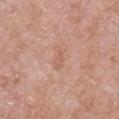Clinical impression:
No biopsy was performed on this lesion — it was imaged during a full skin examination and was not determined to be concerning.
Acquisition and patient details:
The subject is a male in their mid-60s. A region of skin cropped from a whole-body photographic capture, roughly 15 mm wide. Located on the right upper arm.